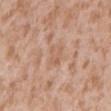Notes:
* follow-up · imaged on a skin check; not biopsied
* image · ~15 mm tile from a whole-body skin photo
* automated metrics · a footprint of about 5 mm² and a symmetry-axis asymmetry near 0.2; an automated nevus-likeness rating near 0 out of 100 and a detector confidence of about 100 out of 100 that the crop contains a lesion
* subject · male, aged approximately 45
* lighting · white-light
* body site · the mid back